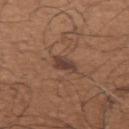<case>
<biopsy_status>not biopsied; imaged during a skin examination</biopsy_status>
<lesion_size>
  <long_diameter_mm_approx>3.0</long_diameter_mm_approx>
</lesion_size>
<lighting>white-light</lighting>
<patient>
  <sex>male</sex>
  <age_approx>65</age_approx>
</patient>
<image>
  <source>total-body photography crop</source>
  <field_of_view_mm>15</field_of_view_mm>
</image>
<site>arm</site>
</case>